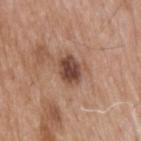An algorithmic analysis of the crop reported an outline eccentricity of about 0.6 (0 = round, 1 = elongated) and two-axis asymmetry of about 0.15. It also reported a border-irregularity rating of about 1.5/10, a color-variation rating of about 4/10, and peripheral color asymmetry of about 1.5. And it measured an automated nevus-likeness rating near 40 out of 100 and a detector confidence of about 100 out of 100 that the crop contains a lesion.
A male subject, roughly 65 years of age.
The lesion is located on the upper back.
This is a white-light tile.
Measured at roughly 3.5 mm in maximum diameter.
This image is a 15 mm lesion crop taken from a total-body photograph.